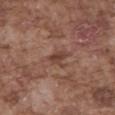This lesion was catalogued during total-body skin photography and was not selected for biopsy.
Approximately 2.5 mm at its widest.
A region of skin cropped from a whole-body photographic capture, roughly 15 mm wide.
Imaged with white-light lighting.
A male subject in their mid-70s.
An algorithmic analysis of the crop reported a footprint of about 3 mm² and an outline eccentricity of about 0.8 (0 = round, 1 = elongated). The software also gave internal color variation of about 1.5 on a 0–10 scale and a peripheral color-asymmetry measure near 0.5.
The lesion is on the abdomen.